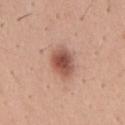From the chest. A male subject approximately 40 years of age. A 15 mm close-up extracted from a 3D total-body photography capture.